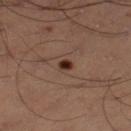{
  "biopsy_status": "not biopsied; imaged during a skin examination",
  "lesion_size": {
    "long_diameter_mm_approx": 1.5
  },
  "site": "left thigh",
  "image": {
    "source": "total-body photography crop",
    "field_of_view_mm": 15
  },
  "patient": {
    "sex": "male",
    "age_approx": 50
  },
  "lighting": "cross-polarized",
  "automated_metrics": {
    "area_mm2_approx": 2.0,
    "eccentricity": 0.55,
    "shape_asymmetry": 0.25,
    "border_irregularity_0_10": 2.0,
    "color_variation_0_10": 2.5,
    "peripheral_color_asymmetry": 1.0
  }
}